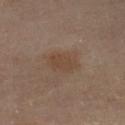Captured during whole-body skin photography for melanoma surveillance; the lesion was not biopsied.
The tile uses cross-polarized illumination.
A female subject approximately 60 years of age.
Located on the leg.
Approximately 3.5 mm at its widest.
A 15 mm close-up extracted from a 3D total-body photography capture.